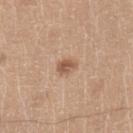Q: Was this lesion biopsied?
A: imaged on a skin check; not biopsied
Q: Where on the body is the lesion?
A: the left lower leg
Q: What lighting was used for the tile?
A: white-light
Q: What is the imaging modality?
A: ~15 mm tile from a whole-body skin photo
Q: What are the patient's age and sex?
A: male, approximately 40 years of age